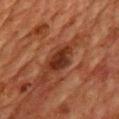* anatomic site: the chest
* tile lighting: cross-polarized illumination
* subject: male, aged 58–62
* acquisition: ~15 mm tile from a whole-body skin photo